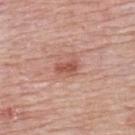Case summary:
- anatomic site · the chest
- image source · ~15 mm tile from a whole-body skin photo
- subject · female, in their mid- to late 60s
- illumination · white-light
- lesion diameter · about 2.5 mm